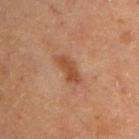Captured during whole-body skin photography for melanoma surveillance; the lesion was not biopsied.
A close-up tile cropped from a whole-body skin photograph, about 15 mm across.
The lesion's longest dimension is about 4 mm.
The lesion is located on the back.
A male subject, roughly 65 years of age.
Imaged with cross-polarized lighting.
The total-body-photography lesion software estimated an area of roughly 5.5 mm². The analysis additionally found a lesion color around L≈48 a*≈24 b*≈34 in CIELAB, roughly 9 lightness units darker than nearby skin, and a normalized lesion–skin contrast near 7.5.